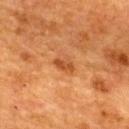No biopsy was performed on this lesion — it was imaged during a full skin examination and was not determined to be concerning. The lesion is located on the upper back. The patient is a female aged 53 to 57. A lesion tile, about 15 mm wide, cut from a 3D total-body photograph. Longest diameter approximately 2.5 mm.